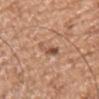The lesion was photographed on a routine skin check and not biopsied; there is no pathology result. On the chest. A male subject, aged approximately 55. A close-up tile cropped from a whole-body skin photograph, about 15 mm across.